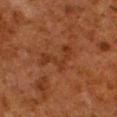Imaged during a routine full-body skin examination; the lesion was not biopsied and no histopathology is available. Captured under cross-polarized illumination. The lesion-visualizer software estimated an area of roughly 8.5 mm² and a shape-asymmetry score of about 0.7 (0 = symmetric). It also reported a mean CIELAB color near L≈28 a*≈21 b*≈28, about 5 CIELAB-L* units darker than the surrounding skin, and a normalized lesion–skin contrast near 5.5. The analysis additionally found a border-irregularity rating of about 8.5/10, internal color variation of about 2 on a 0–10 scale, and radial color variation of about 0.5. Cropped from a whole-body photographic skin survey; the tile spans about 15 mm. The subject is a male aged around 80. The recorded lesion diameter is about 5 mm. The lesion is on the left lower leg.